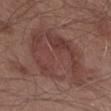{
  "biopsy_status": "not biopsied; imaged during a skin examination",
  "site": "left forearm",
  "image": {
    "source": "total-body photography crop",
    "field_of_view_mm": 15
  },
  "patient": {
    "sex": "male",
    "age_approx": 55
  }
}